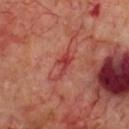The patient is a male about 70 years old. From the chest. A lesion tile, about 15 mm wide, cut from a 3D total-body photograph.